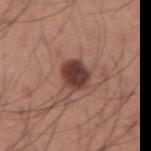Findings:
• notes: imaged on a skin check; not biopsied
• tile lighting: white-light
• site: the arm
• imaging modality: total-body-photography crop, ~15 mm field of view
• patient: male, approximately 35 years of age
• lesion diameter: ~3.5 mm (longest diameter)
• automated lesion analysis: a lesion color around L≈39 a*≈21 b*≈23 in CIELAB and about 15 CIELAB-L* units darker than the surrounding skin; a border-irregularity index near 1.5/10, a color-variation rating of about 3.5/10, and a peripheral color-asymmetry measure near 1; lesion-presence confidence of about 100/100